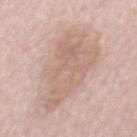follow-up: catalogued during a skin exam; not biopsied
subject: male, approximately 55 years of age
body site: the mid back
image: 15 mm crop, total-body photography